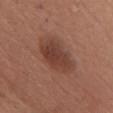automated_metrics:
  cielab_L: 41
  cielab_a: 22
  cielab_b: 27
  vs_skin_darker_L: 10.0
  vs_skin_contrast_norm: 8.0
  border_irregularity_0_10: 2.5
  color_variation_0_10: 3.5
lesion_size:
  long_diameter_mm_approx: 5.5
patient:
  sex: female
  age_approx: 65
image:
  source: total-body photography crop
  field_of_view_mm: 15
site: front of the torso